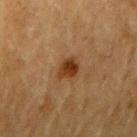{
  "biopsy_status": "not biopsied; imaged during a skin examination",
  "lesion_size": {
    "long_diameter_mm_approx": 2.5
  },
  "patient": {
    "sex": "male",
    "age_approx": "85+"
  },
  "image": {
    "source": "total-body photography crop",
    "field_of_view_mm": 15
  },
  "site": "arm",
  "lighting": "cross-polarized",
  "automated_metrics": {
    "area_mm2_approx": 5.0,
    "shape_asymmetry": 0.25,
    "vs_skin_darker_L": 11.0,
    "vs_skin_contrast_norm": 10.5
  }
}